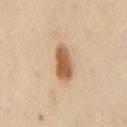Assessment: Imaged during a routine full-body skin examination; the lesion was not biopsied and no histopathology is available. Clinical summary: Located on the front of the torso. A male patient, approximately 50 years of age. The lesion-visualizer software estimated a footprint of about 8 mm² and a shape-asymmetry score of about 0.15 (0 = symmetric). The software also gave a lesion–skin lightness drop of about 14 and a normalized border contrast of about 10. About 4 mm across. A close-up tile cropped from a whole-body skin photograph, about 15 mm across.